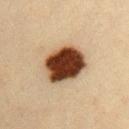This lesion was catalogued during total-body skin photography and was not selected for biopsy. From the front of the torso. A male patient approximately 35 years of age. The recorded lesion diameter is about 5.5 mm. A 15 mm close-up tile from a total-body photography series done for melanoma screening. The lesion-visualizer software estimated an area of roughly 19 mm² and a symmetry-axis asymmetry near 0.1. The software also gave a within-lesion color-variation index near 7.5/10 and radial color variation of about 2. The analysis additionally found a classifier nevus-likeness of about 100/100 and a lesion-detection confidence of about 100/100. Imaged with cross-polarized lighting.